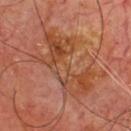<tbp_lesion>
<biopsy_status>not biopsied; imaged during a skin examination</biopsy_status>
<lighting>cross-polarized</lighting>
<lesion_size>
  <long_diameter_mm_approx>8.5</long_diameter_mm_approx>
</lesion_size>
<patient>
  <sex>male</sex>
  <age_approx>65</age_approx>
</patient>
<automated_metrics>
  <cielab_L>46</cielab_L>
  <cielab_a>25</cielab_a>
  <cielab_b>34</cielab_b>
  <vs_skin_darker_L>7.0</vs_skin_darker_L>
  <vs_skin_contrast_norm>6.0</vs_skin_contrast_norm>
</automated_metrics>
<site>chest</site>
<image>
  <source>total-body photography crop</source>
  <field_of_view_mm>15</field_of_view_mm>
</image>
</tbp_lesion>